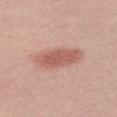Imaged during a routine full-body skin examination; the lesion was not biopsied and no histopathology is available.
The recorded lesion diameter is about 5.5 mm.
On the right thigh.
Captured under white-light illumination.
A female subject in their 20s.
A 15 mm close-up tile from a total-body photography series done for melanoma screening.